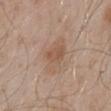Notes:
- notes: imaged on a skin check; not biopsied
- lesion diameter: about 4.5 mm
- site: the mid back
- subject: male, about 55 years old
- acquisition: 15 mm crop, total-body photography